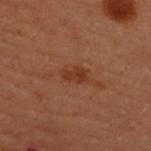Part of a total-body skin-imaging series; this lesion was reviewed on a skin check and was not flagged for biopsy. The tile uses cross-polarized illumination. A female patient aged 48–52. The lesion is on the upper back. The lesion's longest dimension is about 2.5 mm. A roughly 15 mm field-of-view crop from a total-body skin photograph.